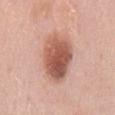Imaged during a routine full-body skin examination; the lesion was not biopsied and no histopathology is available. Captured under white-light illumination. On the mid back. An algorithmic analysis of the crop reported a lesion area of about 20 mm². The software also gave a lesion color around L≈57 a*≈24 b*≈29 in CIELAB. A female subject in their 50s. This image is a 15 mm lesion crop taken from a total-body photograph.